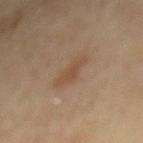- patient — male, aged 83 or over
- body site — the mid back
- tile lighting — cross-polarized
- acquisition — ~15 mm crop, total-body skin-cancer survey
- lesion size — ~4 mm (longest diameter)